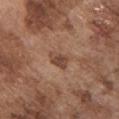acquisition — total-body-photography crop, ~15 mm field of view | location — the chest | lesion diameter — ~2.5 mm (longest diameter) | subject — male, approximately 75 years of age | illumination — white-light illumination.